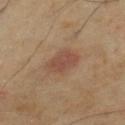Notes:
* notes · total-body-photography surveillance lesion; no biopsy
* anatomic site · the left lower leg
* patient · male, aged approximately 70
* tile lighting · cross-polarized
* image · ~15 mm crop, total-body skin-cancer survey
* automated lesion analysis · a mean CIELAB color near L≈45 a*≈18 b*≈26, about 8 CIELAB-L* units darker than the surrounding skin, and a lesion-to-skin contrast of about 6.5 (normalized; higher = more distinct); a border-irregularity rating of about 2/10, a color-variation rating of about 2.5/10, and radial color variation of about 1
* size · about 4 mm